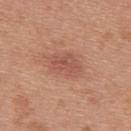Findings:
• notes · total-body-photography surveillance lesion; no biopsy
• patient · female, roughly 40 years of age
• anatomic site · the back
• acquisition · 15 mm crop, total-body photography